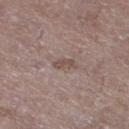* image — ~15 mm tile from a whole-body skin photo
* body site — the left lower leg
* lesion size — ≈2.5 mm
* patient — male, aged around 65
* tile lighting — white-light